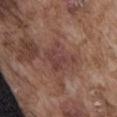No biopsy was performed on this lesion — it was imaged during a full skin examination and was not determined to be concerning.